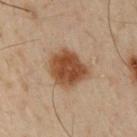Part of a total-body skin-imaging series; this lesion was reviewed on a skin check and was not flagged for biopsy.